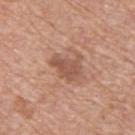The patient is a male in their 60s. Measured at roughly 3.5 mm in maximum diameter. A 15 mm close-up extracted from a 3D total-body photography capture. On the upper back.